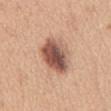{"site": "abdomen", "lesion_size": {"long_diameter_mm_approx": 5.5}, "lighting": "white-light", "patient": {"sex": "female", "age_approx": 30}, "image": {"source": "total-body photography crop", "field_of_view_mm": 15}}